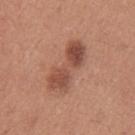Clinical impression: Recorded during total-body skin imaging; not selected for excision or biopsy. Image and clinical context: A 15 mm close-up extracted from a 3D total-body photography capture. Located on the left upper arm. This is a white-light tile. The total-body-photography lesion software estimated an average lesion color of about L≈49 a*≈24 b*≈29 (CIELAB), a lesion–skin lightness drop of about 12, and a lesion-to-skin contrast of about 8 (normalized; higher = more distinct). The analysis additionally found a nevus-likeness score of about 20/100. A female patient, aged 23–27.